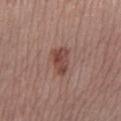The lesion is on the left lower leg. Longest diameter approximately 3.5 mm. This image is a 15 mm lesion crop taken from a total-body photograph. A male subject, about 55 years old. Captured under white-light illumination. The lesion-visualizer software estimated an area of roughly 6.5 mm² and a symmetry-axis asymmetry near 0.3. The software also gave a lesion color around L≈45 a*≈21 b*≈24 in CIELAB, a lesion–skin lightness drop of about 10, and a normalized border contrast of about 8. It also reported border irregularity of about 3 on a 0–10 scale, internal color variation of about 4 on a 0–10 scale, and peripheral color asymmetry of about 1.5.